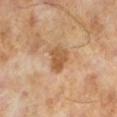follow-up: catalogued during a skin exam; not biopsied
image: ~15 mm crop, total-body skin-cancer survey
subject: male, aged around 65